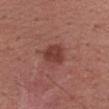Assessment: The lesion was photographed on a routine skin check and not biopsied; there is no pathology result. Clinical summary: Located on the right upper arm. The subject is a female in their mid- to late 50s. A 15 mm close-up tile from a total-body photography series done for melanoma screening. This is a white-light tile. Approximately 3 mm at its widest.